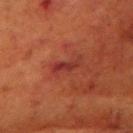This lesion was catalogued during total-body skin photography and was not selected for biopsy. The lesion-visualizer software estimated a lesion color around L≈26 a*≈27 b*≈22 in CIELAB, roughly 6 lightness units darker than nearby skin, and a lesion-to-skin contrast of about 8 (normalized; higher = more distinct). And it measured border irregularity of about 5 on a 0–10 scale, a within-lesion color-variation index near 0/10, and radial color variation of about 0. The analysis additionally found a classifier nevus-likeness of about 0/100 and a lesion-detection confidence of about 95/100. The tile uses cross-polarized illumination. From the head or neck. The patient is a female aged around 80. A close-up tile cropped from a whole-body skin photograph, about 15 mm across.